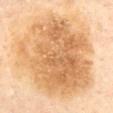Imaged during a routine full-body skin examination; the lesion was not biopsied and no histopathology is available. A female patient, in their 60s. A 15 mm close-up tile from a total-body photography series done for melanoma screening. The lesion is on the back.